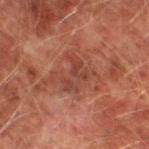No biopsy was performed on this lesion — it was imaged during a full skin examination and was not determined to be concerning.
From the left lower leg.
About 4 mm across.
Cropped from a total-body skin-imaging series; the visible field is about 15 mm.
The lesion-visualizer software estimated roughly 6 lightness units darker than nearby skin and a lesion-to-skin contrast of about 6 (normalized; higher = more distinct). And it measured a lesion-detection confidence of about 100/100.
A male patient, in their mid- to late 70s.